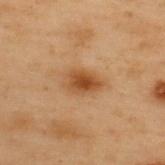patient: male, aged 53–57; image source: ~15 mm tile from a whole-body skin photo; anatomic site: the upper back; illumination: cross-polarized illumination; lesion size: ~4 mm (longest diameter).